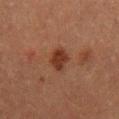Clinical impression:
Imaged during a routine full-body skin examination; the lesion was not biopsied and no histopathology is available.
Background:
A male subject aged 48 to 52. A close-up tile cropped from a whole-body skin photograph, about 15 mm across. The lesion is on the mid back.